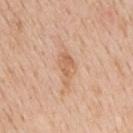No biopsy was performed on this lesion — it was imaged during a full skin examination and was not determined to be concerning.
Located on the mid back.
The lesion-visualizer software estimated an automated nevus-likeness rating near 0 out of 100 and a detector confidence of about 100 out of 100 that the crop contains a lesion.
Approximately 4 mm at its widest.
The subject is a male in their mid-60s.
A 15 mm crop from a total-body photograph taken for skin-cancer surveillance.
Captured under white-light illumination.